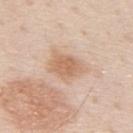The lesion was photographed on a routine skin check and not biopsied; there is no pathology result.
A roughly 15 mm field-of-view crop from a total-body skin photograph.
A male patient, about 80 years old.
The tile uses white-light illumination.
Automated image analysis of the tile measured an outline eccentricity of about 0.7 (0 = round, 1 = elongated) and two-axis asymmetry of about 0.25. And it measured an average lesion color of about L≈65 a*≈19 b*≈32 (CIELAB) and a normalized lesion–skin contrast near 7. And it measured a classifier nevus-likeness of about 5/100 and a detector confidence of about 100 out of 100 that the crop contains a lesion.
Measured at roughly 4.5 mm in maximum diameter.
From the mid back.